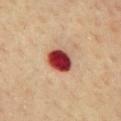Recorded during total-body skin imaging; not selected for excision or biopsy. The subject is a male in their 60s. Cropped from a whole-body photographic skin survey; the tile spans about 15 mm. Automated image analysis of the tile measured a footprint of about 8 mm², an eccentricity of roughly 0.5, and a shape-asymmetry score of about 0.15 (0 = symmetric). The software also gave a border-irregularity index near 1.5/10, internal color variation of about 6.5 on a 0–10 scale, and radial color variation of about 2. The software also gave a classifier nevus-likeness of about 0/100 and a lesion-detection confidence of about 100/100. The lesion is on the chest. Captured under cross-polarized illumination. The recorded lesion diameter is about 3 mm.